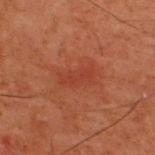{"biopsy_status": "not biopsied; imaged during a skin examination", "patient": {"sex": "male", "age_approx": 60}, "site": "upper back", "automated_metrics": {"area_mm2_approx": 6.0, "eccentricity": 0.9, "shape_asymmetry": 0.25, "cielab_L": 31, "cielab_a": 25, "cielab_b": 27, "vs_skin_darker_L": 5.0, "vs_skin_contrast_norm": 4.5, "nevus_likeness_0_100": 15}, "image": {"source": "total-body photography crop", "field_of_view_mm": 15}, "lighting": "cross-polarized"}